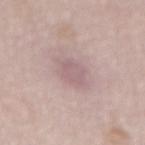biopsy status: no biopsy performed (imaged during a skin exam).